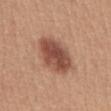Q: Was this lesion biopsied?
A: no biopsy performed (imaged during a skin exam)
Q: How was the tile lit?
A: white-light illumination
Q: Patient demographics?
A: female, in their 40s
Q: What did automated image analysis measure?
A: about 14 CIELAB-L* units darker than the surrounding skin and a normalized lesion–skin contrast near 10
Q: What is the imaging modality?
A: 15 mm crop, total-body photography
Q: What is the anatomic site?
A: the abdomen
Q: Lesion size?
A: ~5.5 mm (longest diameter)